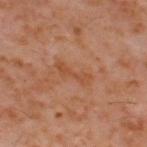follow-up — total-body-photography surveillance lesion; no biopsy
lighting — cross-polarized
patient — male, aged around 60
site — the back
image source — total-body-photography crop, ~15 mm field of view
lesion diameter — ≈4.5 mm